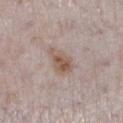Background:
The tile uses white-light illumination. The lesion's longest dimension is about 4 mm. A 15 mm close-up tile from a total-body photography series done for melanoma screening. From the left lower leg. The subject is a female roughly 30 years of age. An algorithmic analysis of the crop reported a mean CIELAB color near L≈55 a*≈16 b*≈25, about 10 CIELAB-L* units darker than the surrounding skin, and a normalized border contrast of about 8. It also reported a within-lesion color-variation index near 4/10 and peripheral color asymmetry of about 1.5.